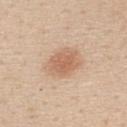| key | value |
|---|---|
| biopsy status | imaged on a skin check; not biopsied |
| subject | female, approximately 45 years of age |
| tile lighting | white-light illumination |
| automated metrics | a lesion color around L≈64 a*≈19 b*≈32 in CIELAB, about 10 CIELAB-L* units darker than the surrounding skin, and a normalized border contrast of about 6.5; a border-irregularity index near 1.5/10, a color-variation rating of about 2.5/10, and radial color variation of about 0.5; a classifier nevus-likeness of about 90/100 |
| imaging modality | ~15 mm crop, total-body skin-cancer survey |
| body site | the upper back |
| size | about 4.5 mm |